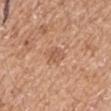biopsy_status: not biopsied; imaged during a skin examination
lesion_size:
  long_diameter_mm_approx: 2.5
image:
  source: total-body photography crop
  field_of_view_mm: 15
lighting: white-light
patient:
  sex: male
  age_approx: 55
automated_metrics:
  area_mm2_approx: 4.5
  eccentricity: 0.6
  shape_asymmetry: 0.2
  cielab_L: 57
  cielab_a: 21
  cielab_b: 33
  vs_skin_darker_L: 8.0
  vs_skin_contrast_norm: 5.0
  border_irregularity_0_10: 2.0
  color_variation_0_10: 2.0
  peripheral_color_asymmetry: 0.5
  lesion_detection_confidence_0_100: 100
site: right upper arm